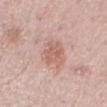Case summary:
• follow-up — no biopsy performed (imaged during a skin exam)
• location — the abdomen
• patient — male, aged approximately 70
• imaging modality — ~15 mm tile from a whole-body skin photo